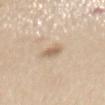biopsy status: imaged on a skin check; not biopsied | body site: the mid back | subject: female, aged 38–42 | image: total-body-photography crop, ~15 mm field of view | image-analysis metrics: a footprint of about 4 mm², an eccentricity of roughly 0.75, and a shape-asymmetry score of about 0.25 (0 = symmetric); a lesion color around L≈64 a*≈14 b*≈30 in CIELAB, roughly 10 lightness units darker than nearby skin, and a normalized lesion–skin contrast near 6.5; a classifier nevus-likeness of about 85/100 and a detector confidence of about 100 out of 100 that the crop contains a lesion | lesion diameter: about 2.5 mm | illumination: white-light.